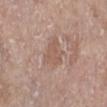The lesion was photographed on a routine skin check and not biopsied; there is no pathology result.